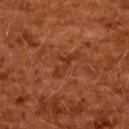Background:
This image is a 15 mm lesion crop taken from a total-body photograph. An algorithmic analysis of the crop reported a shape eccentricity near 0.9 and a symmetry-axis asymmetry near 0.5. And it measured a mean CIELAB color near L≈28 a*≈22 b*≈29 and a lesion-to-skin contrast of about 5.5 (normalized; higher = more distinct). The analysis additionally found a border-irregularity rating of about 7/10, internal color variation of about 0 on a 0–10 scale, and radial color variation of about 0. It also reported a nevus-likeness score of about 0/100. Imaged with cross-polarized lighting. The lesion's longest dimension is about 4 mm. The lesion is on the back. The patient is a female aged 48 to 52.